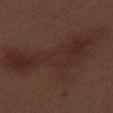No biopsy was performed on this lesion — it was imaged during a full skin examination and was not determined to be concerning.
A male patient, aged around 70.
The recorded lesion diameter is about 15 mm.
The lesion is on the right thigh.
Automated tile analysis of the lesion measured an area of roughly 55 mm² and a shape-asymmetry score of about 0.55 (0 = symmetric). The analysis additionally found an average lesion color of about L≈28 a*≈18 b*≈21 (CIELAB), roughly 5 lightness units darker than nearby skin, and a normalized border contrast of about 6. The analysis additionally found a nevus-likeness score of about 0/100 and a detector confidence of about 100 out of 100 that the crop contains a lesion.
A 15 mm close-up tile from a total-body photography series done for melanoma screening.